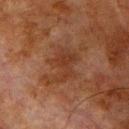Clinical impression:
The lesion was photographed on a routine skin check and not biopsied; there is no pathology result.
Context:
On the chest. The tile uses cross-polarized illumination. The lesion-visualizer software estimated a normalized lesion–skin contrast near 5.5. And it measured a color-variation rating of about 3/10 and peripheral color asymmetry of about 1. And it measured an automated nevus-likeness rating near 0 out of 100 and a lesion-detection confidence of about 100/100. Measured at roughly 6 mm in maximum diameter. A 15 mm crop from a total-body photograph taken for skin-cancer surveillance. A male patient about 80 years old.